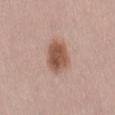<tbp_lesion>
<biopsy_status>not biopsied; imaged during a skin examination</biopsy_status>
<image>
  <source>total-body photography crop</source>
  <field_of_view_mm>15</field_of_view_mm>
</image>
<site>mid back</site>
<patient>
  <sex>female</sex>
  <age_approx>30</age_approx>
</patient>
</tbp_lesion>